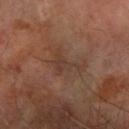This lesion was catalogued during total-body skin photography and was not selected for biopsy. The lesion is located on the right forearm. A roughly 15 mm field-of-view crop from a total-body skin photograph. Imaged with cross-polarized lighting. The patient is a male in their mid-60s. Measured at roughly 3.5 mm in maximum diameter.